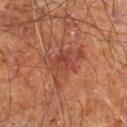Part of a total-body skin-imaging series; this lesion was reviewed on a skin check and was not flagged for biopsy.